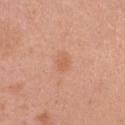A female patient, approximately 40 years of age. The lesion is on the right upper arm. This image is a 15 mm lesion crop taken from a total-body photograph.